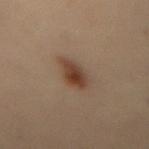{"biopsy_status": "not biopsied; imaged during a skin examination", "automated_metrics": {"area_mm2_approx": 6.5, "eccentricity": 0.9, "shape_asymmetry": 0.2, "cielab_L": 31, "cielab_a": 15, "cielab_b": 23, "vs_skin_contrast_norm": 9.5, "nevus_likeness_0_100": 100, "lesion_detection_confidence_0_100": 100}, "image": {"source": "total-body photography crop", "field_of_view_mm": 15}, "lesion_size": {"long_diameter_mm_approx": 4.0}, "site": "lower back", "patient": {"sex": "female", "age_approx": 30}, "lighting": "cross-polarized"}